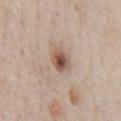{
  "biopsy_status": "not biopsied; imaged during a skin examination",
  "automated_metrics": {
    "area_mm2_approx": 5.5,
    "eccentricity": 0.45,
    "border_irregularity_0_10": 1.5,
    "color_variation_0_10": 7.5,
    "peripheral_color_asymmetry": 2.5,
    "lesion_detection_confidence_0_100": 100
  },
  "lesion_size": {
    "long_diameter_mm_approx": 3.0
  },
  "site": "front of the torso",
  "lighting": "white-light",
  "patient": {
    "sex": "male",
    "age_approx": 60
  },
  "image": {
    "source": "total-body photography crop",
    "field_of_view_mm": 15
  }
}